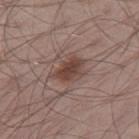Assessment: Part of a total-body skin-imaging series; this lesion was reviewed on a skin check and was not flagged for biopsy. Context: The recorded lesion diameter is about 4 mm. The lesion is located on the right thigh. The tile uses white-light illumination. A male patient aged approximately 55. A close-up tile cropped from a whole-body skin photograph, about 15 mm across.